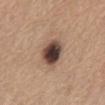follow-up = catalogued during a skin exam; not biopsied | size = ~4 mm (longest diameter) | lighting = white-light | site = the chest | imaging modality = 15 mm crop, total-body photography | subject = female, aged approximately 55.